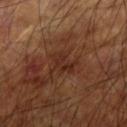No biopsy was performed on this lesion — it was imaged during a full skin examination and was not determined to be concerning. Captured under cross-polarized illumination. The lesion is on the arm. The subject is a male about 60 years old. The lesion's longest dimension is about 3.5 mm. A 15 mm close-up extracted from a 3D total-body photography capture.